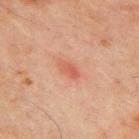Clinical impression: Recorded during total-body skin imaging; not selected for excision or biopsy. Image and clinical context: About 3 mm across. Captured under cross-polarized illumination. A male patient approximately 70 years of age. A 15 mm close-up tile from a total-body photography series done for melanoma screening. Located on the chest.